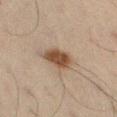Notes:
– anatomic site · the left thigh
– subject · male, about 65 years old
– acquisition · total-body-photography crop, ~15 mm field of view
– image-analysis metrics · an outline eccentricity of about 0.8 (0 = round, 1 = elongated) and a symmetry-axis asymmetry near 0.2; a border-irregularity rating of about 2/10 and a color-variation rating of about 2/10; a nevus-likeness score of about 95/100 and a detector confidence of about 100 out of 100 that the crop contains a lesion
– size · ≈4 mm
– lighting · cross-polarized illumination